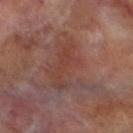Captured during whole-body skin photography for melanoma surveillance; the lesion was not biopsied.